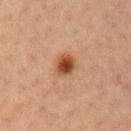No biopsy was performed on this lesion — it was imaged during a full skin examination and was not determined to be concerning.